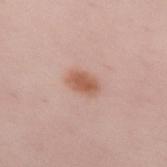Imaged during a routine full-body skin examination; the lesion was not biopsied and no histopathology is available. A region of skin cropped from a whole-body photographic capture, roughly 15 mm wide. A female subject in their mid-20s. Located on the left forearm.